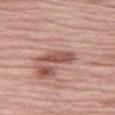The lesion was tiled from a total-body skin photograph and was not biopsied.